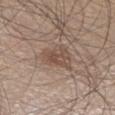The total-body-photography lesion software estimated an outline eccentricity of about 0.65 (0 = round, 1 = elongated) and two-axis asymmetry of about 0.3. It also reported border irregularity of about 3.5 on a 0–10 scale and a within-lesion color-variation index near 3/10. The software also gave an automated nevus-likeness rating near 15 out of 100 and lesion-presence confidence of about 100/100. The subject is a male about 45 years old. Measured at roughly 4.5 mm in maximum diameter. The lesion is on the right lower leg. Captured under white-light illumination. A roughly 15 mm field-of-view crop from a total-body skin photograph.